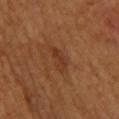The lesion was tiled from a total-body skin photograph and was not biopsied. A 15 mm close-up extracted from a 3D total-body photography capture. A male patient, about 65 years old. The lesion is on the back.